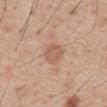follow-up: imaged on a skin check; not biopsied.